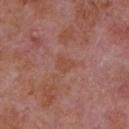* notes — catalogued during a skin exam; not biopsied
* imaging modality — total-body-photography crop, ~15 mm field of view
* site — the front of the torso
* patient — male, aged around 65